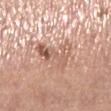Image and clinical context:
Approximately 5 mm at its widest. A roughly 15 mm field-of-view crop from a total-body skin photograph. Imaged with white-light lighting. The lesion is on the left lower leg. A female patient approximately 65 years of age.